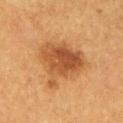Assessment:
Imaged during a routine full-body skin examination; the lesion was not biopsied and no histopathology is available.
Context:
Captured under cross-polarized illumination. From the left lower leg. This image is a 15 mm lesion crop taken from a total-body photograph. The recorded lesion diameter is about 7 mm. The lesion-visualizer software estimated an area of roughly 23 mm² and two-axis asymmetry of about 0.3. The analysis additionally found an average lesion color of about L≈43 a*≈21 b*≈34 (CIELAB), a lesion–skin lightness drop of about 10, and a normalized lesion–skin contrast near 8. It also reported a border-irregularity rating of about 3.5/10, internal color variation of about 5 on a 0–10 scale, and a peripheral color-asymmetry measure near 1.5. The analysis additionally found an automated nevus-likeness rating near 80 out of 100 and a lesion-detection confidence of about 100/100. A female patient approximately 40 years of age.